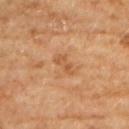Q: Was this lesion biopsied?
A: catalogued during a skin exam; not biopsied
Q: Illumination type?
A: cross-polarized illumination
Q: How large is the lesion?
A: ≈3 mm
Q: What are the patient's age and sex?
A: female, in their 60s
Q: What did automated image analysis measure?
A: a lesion area of about 3.5 mm², an outline eccentricity of about 0.9 (0 = round, 1 = elongated), and two-axis asymmetry of about 0.25; border irregularity of about 3.5 on a 0–10 scale, a within-lesion color-variation index near 0/10, and peripheral color asymmetry of about 0
Q: Where on the body is the lesion?
A: the upper back
Q: How was this image acquired?
A: 15 mm crop, total-body photography